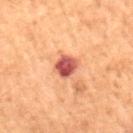notes = imaged on a skin check; not biopsied | body site = the chest | imaging modality = total-body-photography crop, ~15 mm field of view | patient = female, aged 78 to 82.